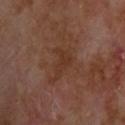<tbp_lesion>
  <biopsy_status>not biopsied; imaged during a skin examination</biopsy_status>
  <lighting>cross-polarized</lighting>
  <patient>
    <sex>male</sex>
    <age_approx>70</age_approx>
  </patient>
  <image>
    <source>total-body photography crop</source>
    <field_of_view_mm>15</field_of_view_mm>
  </image>
  <site>back</site>
  <automated_metrics>
    <area_mm2_approx>5.5</area_mm2_approx>
    <shape_asymmetry>0.5</shape_asymmetry>
    <cielab_L>32</cielab_L>
    <cielab_a>19</cielab_a>
    <cielab_b>27</cielab_b>
    <vs_skin_darker_L>5.0</vs_skin_darker_L>
    <vs_skin_contrast_norm>6.0</vs_skin_contrast_norm>
    <peripheral_color_asymmetry>0.5</peripheral_color_asymmetry>
  </automated_metrics>
</tbp_lesion>